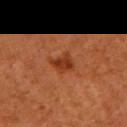Assessment: This lesion was catalogued during total-body skin photography and was not selected for biopsy. Acquisition and patient details: Measured at roughly 3 mm in maximum diameter. This image is a 15 mm lesion crop taken from a total-body photograph. The lesion is located on the left upper arm. A female subject, in their mid-60s.